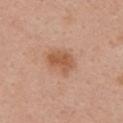Findings:
• follow-up — total-body-photography surveillance lesion; no biopsy